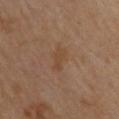Assessment: The lesion was tiled from a total-body skin photograph and was not biopsied. Acquisition and patient details: A close-up tile cropped from a whole-body skin photograph, about 15 mm across. The recorded lesion diameter is about 3 mm. Imaged with cross-polarized lighting. The subject is a male roughly 55 years of age. From the back.